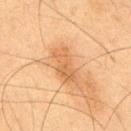Findings:
– notes — catalogued during a skin exam; not biopsied
– image source — ~15 mm crop, total-body skin-cancer survey
– tile lighting — cross-polarized illumination
– anatomic site — the back
– automated metrics — a lesion area of about 8 mm², a shape eccentricity near 0.9, and two-axis asymmetry of about 0.3; a lesion color around L≈49 a*≈19 b*≈34 in CIELAB and about 7 CIELAB-L* units darker than the surrounding skin; a classifier nevus-likeness of about 0/100 and a detector confidence of about 100 out of 100 that the crop contains a lesion
– patient — male, aged 33–37